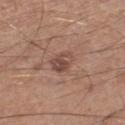No biopsy was performed on this lesion — it was imaged during a full skin examination and was not determined to be concerning.
About 3 mm across.
Cropped from a whole-body photographic skin survey; the tile spans about 15 mm.
The patient is a male aged around 60.
This is a white-light tile.
The lesion is located on the left lower leg.